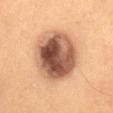notes=imaged on a skin check; not biopsied
diameter=≈7 mm
image source=15 mm crop, total-body photography
site=the abdomen
lighting=cross-polarized
subject=female, roughly 50 years of age